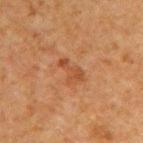Imaged during a routine full-body skin examination; the lesion was not biopsied and no histopathology is available. From the left arm. The subject is a male approximately 60 years of age. Imaged with cross-polarized lighting. A roughly 15 mm field-of-view crop from a total-body skin photograph.